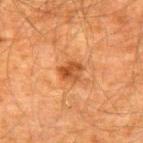The lesion was photographed on a routine skin check and not biopsied; there is no pathology result. A lesion tile, about 15 mm wide, cut from a 3D total-body photograph. The tile uses cross-polarized illumination. From the upper back. About 2.5 mm across. A male patient, roughly 60 years of age. Automated image analysis of the tile measured an area of roughly 5 mm², a shape eccentricity near 0.55, and a symmetry-axis asymmetry near 0.25. The analysis additionally found an average lesion color of about L≈40 a*≈24 b*≈35 (CIELAB), about 10 CIELAB-L* units darker than the surrounding skin, and a normalized border contrast of about 8. The software also gave a border-irregularity rating of about 2.5/10, a color-variation rating of about 4.5/10, and peripheral color asymmetry of about 1.5. The software also gave a nevus-likeness score of about 55/100 and lesion-presence confidence of about 100/100.